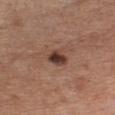The lesion was photographed on a routine skin check and not biopsied; there is no pathology result. Imaged with white-light lighting. Cropped from a total-body skin-imaging series; the visible field is about 15 mm. The patient is a female in their mid- to late 70s. The lesion is on the chest. The lesion-visualizer software estimated a lesion area of about 5 mm², an eccentricity of roughly 0.75, and two-axis asymmetry of about 0.25. The software also gave a classifier nevus-likeness of about 80/100 and a lesion-detection confidence of about 100/100. The lesion's longest dimension is about 3 mm.